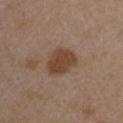Part of a total-body skin-imaging series; this lesion was reviewed on a skin check and was not flagged for biopsy. The patient is a male aged 48 to 52. This image is a 15 mm lesion crop taken from a total-body photograph. Automated tile analysis of the lesion measured border irregularity of about 2 on a 0–10 scale, internal color variation of about 2 on a 0–10 scale, and peripheral color asymmetry of about 0.5. And it measured a classifier nevus-likeness of about 95/100. The tile uses white-light illumination. The lesion is on the arm.